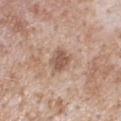biopsy_status: not biopsied; imaged during a skin examination
automated_metrics:
  border_irregularity_0_10: 2.5
  color_variation_0_10: 2.5
site: chest
patient:
  sex: male
  age_approx: 65
lighting: white-light
lesion_size:
  long_diameter_mm_approx: 3.0
image:
  source: total-body photography crop
  field_of_view_mm: 15